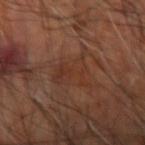{"biopsy_status": "not biopsied; imaged during a skin examination", "lighting": "cross-polarized", "patient": {"age_approx": 65}, "site": "right upper arm", "lesion_size": {"long_diameter_mm_approx": 5.0}, "image": {"source": "total-body photography crop", "field_of_view_mm": 15}, "automated_metrics": {"cielab_L": 33, "cielab_a": 21, "cielab_b": 27, "vs_skin_contrast_norm": 5.5}}